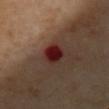* workup · catalogued during a skin exam; not biopsied
* subject · female, aged around 55
* site · the chest
* lesion diameter · about 2.5 mm
* image · 15 mm crop, total-body photography